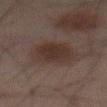Recorded during total-body skin imaging; not selected for excision or biopsy. The patient is a male aged 63 to 67. The tile uses cross-polarized illumination. A 15 mm crop from a total-body photograph taken for skin-cancer surveillance. On the mid back.